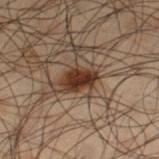Part of a total-body skin-imaging series; this lesion was reviewed on a skin check and was not flagged for biopsy.
Captured under cross-polarized illumination.
A male patient roughly 50 years of age.
A region of skin cropped from a whole-body photographic capture, roughly 15 mm wide.
The lesion is located on the left thigh.
Longest diameter approximately 3.5 mm.
The total-body-photography lesion software estimated an area of roughly 5.5 mm², a shape eccentricity near 0.8, and a symmetry-axis asymmetry near 0.25. And it measured a lesion color around L≈24 a*≈15 b*≈22 in CIELAB, about 12 CIELAB-L* units darker than the surrounding skin, and a lesion-to-skin contrast of about 13 (normalized; higher = more distinct). And it measured a border-irregularity index near 2.5/10, internal color variation of about 2.5 on a 0–10 scale, and radial color variation of about 1.